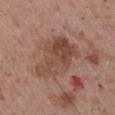Clinical summary:
A 15 mm close-up extracted from a 3D total-body photography capture. A male patient in their 70s. The lesion is on the left upper arm. Captured under white-light illumination.